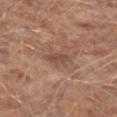Notes:
* follow-up: no biopsy performed (imaged during a skin exam)
* imaging modality: 15 mm crop, total-body photography
* subject: male, approximately 60 years of age
* lesion diameter: ~2.5 mm (longest diameter)
* TBP lesion metrics: an average lesion color of about L≈48 a*≈20 b*≈27 (CIELAB), roughly 8 lightness units darker than nearby skin, and a normalized lesion–skin contrast near 6; a classifier nevus-likeness of about 0/100 and lesion-presence confidence of about 100/100
* location: the right upper arm